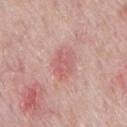follow-up: imaged on a skin check; not biopsied | lesion size: about 3 mm | imaging modality: total-body-photography crop, ~15 mm field of view | body site: the front of the torso | patient: male, aged 63 to 67 | lighting: white-light illumination.